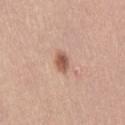Captured during whole-body skin photography for melanoma surveillance; the lesion was not biopsied. The lesion's longest dimension is about 2.5 mm. A female patient aged approximately 45. From the right thigh. The lesion-visualizer software estimated a nevus-likeness score of about 95/100 and lesion-presence confidence of about 100/100. This is a white-light tile. Cropped from a total-body skin-imaging series; the visible field is about 15 mm.